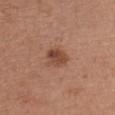Notes:
- biopsy status: total-body-photography surveillance lesion; no biopsy
- patient: female, in their mid-60s
- imaging modality: ~15 mm crop, total-body skin-cancer survey
- image-analysis metrics: an automated nevus-likeness rating near 90 out of 100 and lesion-presence confidence of about 100/100
- anatomic site: the chest
- tile lighting: white-light illumination
- diameter: ~3 mm (longest diameter)